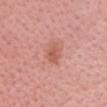This lesion was catalogued during total-body skin photography and was not selected for biopsy. A female subject, aged approximately 40. From the chest. A roughly 15 mm field-of-view crop from a total-body skin photograph.